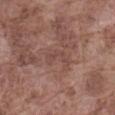Notes:
• notes · catalogued during a skin exam; not biopsied
• image source · total-body-photography crop, ~15 mm field of view
• site · the abdomen
• subject · male, in their mid-70s